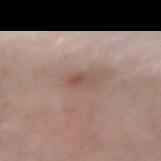subject: male, aged 58–62; automated lesion analysis: a border-irregularity rating of about 2.5/10, internal color variation of about 2.5 on a 0–10 scale, and radial color variation of about 1; acquisition: 15 mm crop, total-body photography; anatomic site: the left forearm; tile lighting: white-light illumination; lesion size: about 2.5 mm.